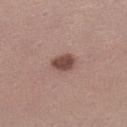* workup · no biopsy performed (imaged during a skin exam)
* automated metrics · a border-irregularity index near 1.5/10 and a within-lesion color-variation index near 2/10; a nevus-likeness score of about 95/100 and a lesion-detection confidence of about 100/100
* imaging modality · ~15 mm tile from a whole-body skin photo
* location · the left lower leg
* diameter · ~3 mm (longest diameter)
* patient · female, aged around 30
* illumination · white-light